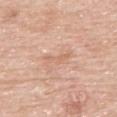Clinical impression:
The lesion was photographed on a routine skin check and not biopsied; there is no pathology result.
Acquisition and patient details:
Longest diameter approximately 3.5 mm. This is a white-light tile. A close-up tile cropped from a whole-body skin photograph, about 15 mm across. The lesion is located on the mid back. The patient is a male roughly 80 years of age.